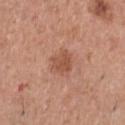biopsy status: total-body-photography surveillance lesion; no biopsy | subject: male, aged 43 to 47 | lesion diameter: ~2.5 mm (longest diameter) | image-analysis metrics: a mean CIELAB color near L≈52 a*≈24 b*≈31, a lesion–skin lightness drop of about 9, and a normalized border contrast of about 6.5; border irregularity of about 2.5 on a 0–10 scale, internal color variation of about 2.5 on a 0–10 scale, and peripheral color asymmetry of about 1; a classifier nevus-likeness of about 35/100 | location: the chest | lighting: white-light illumination | image source: 15 mm crop, total-body photography.